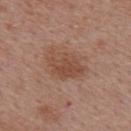{
  "biopsy_status": "not biopsied; imaged during a skin examination",
  "lighting": "white-light",
  "patient": {
    "sex": "female",
    "age_approx": 40
  },
  "lesion_size": {
    "long_diameter_mm_approx": 5.0
  },
  "image": {
    "source": "total-body photography crop",
    "field_of_view_mm": 15
  },
  "site": "upper back"
}